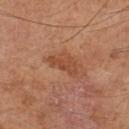Q: Is there a histopathology result?
A: no biopsy performed (imaged during a skin exam)
Q: What is the lesion's diameter?
A: ~4 mm (longest diameter)
Q: What is the imaging modality?
A: ~15 mm tile from a whole-body skin photo
Q: Automated lesion metrics?
A: a mean CIELAB color near L≈45 a*≈24 b*≈32, a lesion–skin lightness drop of about 7, and a normalized border contrast of about 6
Q: Where on the body is the lesion?
A: the left lower leg
Q: Who is the patient?
A: male, aged approximately 65
Q: What lighting was used for the tile?
A: cross-polarized illumination